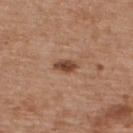acquisition=15 mm crop, total-body photography; diameter=~2.5 mm (longest diameter); automated lesion analysis=a color-variation rating of about 4/10 and a peripheral color-asymmetry measure near 1; lighting=white-light; location=the upper back; patient=male, aged 63–67.